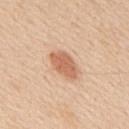workup: no biopsy performed (imaged during a skin exam)
subject: male, roughly 60 years of age
automated lesion analysis: a footprint of about 8 mm², an outline eccentricity of about 0.85 (0 = round, 1 = elongated), and a shape-asymmetry score of about 0.15 (0 = symmetric); a lesion color around L≈63 a*≈23 b*≈34 in CIELAB, a lesion–skin lightness drop of about 12, and a normalized lesion–skin contrast near 7.5; border irregularity of about 2 on a 0–10 scale and a peripheral color-asymmetry measure near 0.5; a classifier nevus-likeness of about 95/100
illumination: white-light
acquisition: total-body-photography crop, ~15 mm field of view
lesion size: about 4.5 mm
body site: the upper back